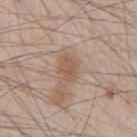Assessment:
No biopsy was performed on this lesion — it was imaged during a full skin examination and was not determined to be concerning.
Clinical summary:
About 3.5 mm across. Cropped from a whole-body photographic skin survey; the tile spans about 15 mm. A male patient, in their mid- to late 40s. The lesion is on the chest.